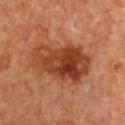This lesion was catalogued during total-body skin photography and was not selected for biopsy. Approximately 7.5 mm at its widest. From the chest. A region of skin cropped from a whole-body photographic capture, roughly 15 mm wide. This is a cross-polarized tile. The subject is a female aged approximately 55. An algorithmic analysis of the crop reported a lesion area of about 25 mm², an eccentricity of roughly 0.8, and a shape-asymmetry score of about 0.25 (0 = symmetric). And it measured a mean CIELAB color near L≈33 a*≈23 b*≈29.